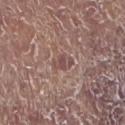The lesion was photographed on a routine skin check and not biopsied; there is no pathology result. An algorithmic analysis of the crop reported an area of roughly 4.5 mm², a shape eccentricity near 0.75, and a symmetry-axis asymmetry near 0.25. The analysis additionally found a border-irregularity rating of about 2.5/10 and a within-lesion color-variation index near 2.5/10. And it measured an automated nevus-likeness rating near 0 out of 100 and a detector confidence of about 55 out of 100 that the crop contains a lesion. Captured under white-light illumination. On the left lower leg. A male patient, aged around 80. A 15 mm close-up tile from a total-body photography series done for melanoma screening. Measured at roughly 2.5 mm in maximum diameter.